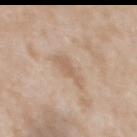Notes:
* site: the upper back
* image-analysis metrics: an area of roughly 5 mm², an eccentricity of roughly 0.85, and two-axis asymmetry of about 0.4
* acquisition: total-body-photography crop, ~15 mm field of view
* patient: female, about 40 years old
* diameter: about 3.5 mm